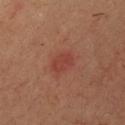  biopsy_status: not biopsied; imaged during a skin examination
  image:
    source: total-body photography crop
    field_of_view_mm: 15
  patient:
    sex: male
    age_approx: 35
  site: front of the torso
  lighting: cross-polarized